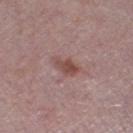On the right lower leg. A male subject, aged 73–77. Imaged with white-light lighting. Cropped from a total-body skin-imaging series; the visible field is about 15 mm. About 3 mm across.